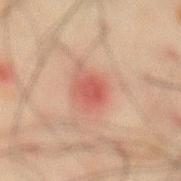Findings:
• follow-up — imaged on a skin check; not biopsied
• subject — male, aged 58–62
• lighting — cross-polarized illumination
• acquisition — total-body-photography crop, ~15 mm field of view
• TBP lesion metrics — a mean CIELAB color near L≈43 a*≈25 b*≈24 and a lesion–skin lightness drop of about 8; a border-irregularity index near 2/10 and internal color variation of about 2 on a 0–10 scale
• location — the abdomen
• size — ~3 mm (longest diameter)